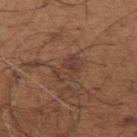size — ≈3.5 mm
imaging modality — 15 mm crop, total-body photography
subject — male, aged around 65
automated metrics — an average lesion color of about L≈39 a*≈18 b*≈26 (CIELAB) and roughly 6 lightness units darker than nearby skin; a classifier nevus-likeness of about 0/100 and lesion-presence confidence of about 60/100
location — the arm